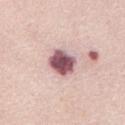The lesion was tiled from a total-body skin photograph and was not biopsied. On the abdomen. Imaged with white-light lighting. Measured at roughly 3.5 mm in maximum diameter. A close-up tile cropped from a whole-body skin photograph, about 15 mm across. Automated tile analysis of the lesion measured a lesion area of about 8.5 mm², an eccentricity of roughly 0.5, and a shape-asymmetry score of about 0.15 (0 = symmetric). It also reported an automated nevus-likeness rating near 10 out of 100 and a lesion-detection confidence of about 100/100. The patient is a female aged approximately 65.